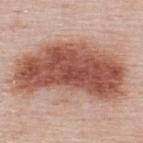Captured during whole-body skin photography for melanoma surveillance; the lesion was not biopsied.
This is a white-light tile.
The patient is a female aged approximately 50.
About 12.5 mm across.
The lesion is located on the back.
The total-body-photography lesion software estimated an average lesion color of about L≈54 a*≈24 b*≈28 (CIELAB) and roughly 17 lightness units darker than nearby skin. And it measured internal color variation of about 7.5 on a 0–10 scale and radial color variation of about 2.5.
This image is a 15 mm lesion crop taken from a total-body photograph.